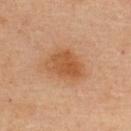Impression:
No biopsy was performed on this lesion — it was imaged during a full skin examination and was not determined to be concerning.
Image and clinical context:
Cropped from a whole-body photographic skin survey; the tile spans about 15 mm. The patient is a female approximately 45 years of age. On the upper back.